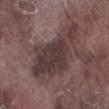biopsy status: no biopsy performed (imaged during a skin exam) | location: the leg | lesion size: ~9.5 mm (longest diameter) | imaging modality: ~15 mm tile from a whole-body skin photo | illumination: white-light illumination | subject: male, about 75 years old | automated metrics: a lesion–skin lightness drop of about 9; border irregularity of about 4.5 on a 0–10 scale and a within-lesion color-variation index near 4.5/10; a detector confidence of about 75 out of 100 that the crop contains a lesion.